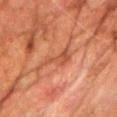  biopsy_status: not biopsied; imaged during a skin examination